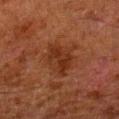| field | value |
|---|---|
| notes | catalogued during a skin exam; not biopsied |
| subject | male, aged around 80 |
| image source | ~15 mm tile from a whole-body skin photo |
| anatomic site | the left lower leg |
| lighting | cross-polarized |